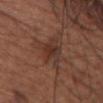<tbp_lesion>
<biopsy_status>not biopsied; imaged during a skin examination</biopsy_status>
<patient>
  <sex>female</sex>
  <age_approx>75</age_approx>
</patient>
<site>head or neck</site>
<image>
  <source>total-body photography crop</source>
  <field_of_view_mm>15</field_of_view_mm>
</image>
<lighting>white-light</lighting>
</tbp_lesion>